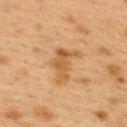Part of a total-body skin-imaging series; this lesion was reviewed on a skin check and was not flagged for biopsy. A female patient, aged 38–42. The lesion is on the upper back. A 15 mm close-up extracted from a 3D total-body photography capture.